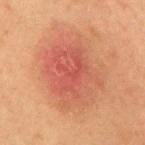follow-up=no biopsy performed (imaged during a skin exam)
patient=female, roughly 60 years of age
lighting=cross-polarized illumination
imaging modality=total-body-photography crop, ~15 mm field of view
automated lesion analysis=an area of roughly 43 mm² and an eccentricity of roughly 0.65; a border-irregularity index near 2.5/10, a within-lesion color-variation index near 6/10, and a peripheral color-asymmetry measure near 2
size=≈8.5 mm
body site=the mid back